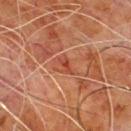Recorded during total-body skin imaging; not selected for excision or biopsy. Imaged with cross-polarized lighting. Cropped from a whole-body photographic skin survey; the tile spans about 15 mm. The lesion is located on the chest. A male subject aged 78–82.